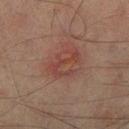{
  "automated_metrics": {
    "area_mm2_approx": 6.5,
    "cielab_L": 35,
    "cielab_a": 21,
    "cielab_b": 22,
    "vs_skin_darker_L": 6.0,
    "vs_skin_contrast_norm": 5.5,
    "border_irregularity_0_10": 8.5,
    "peripheral_color_asymmetry": 0.0
  },
  "image": {
    "source": "total-body photography crop",
    "field_of_view_mm": 15
  },
  "site": "right lower leg",
  "lesion_size": {
    "long_diameter_mm_approx": 4.0
  },
  "patient": {
    "sex": "male",
    "age_approx": 75
  }
}